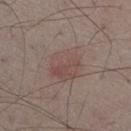{
  "biopsy_status": "not biopsied; imaged during a skin examination",
  "lighting": "white-light",
  "image": {
    "source": "total-body photography crop",
    "field_of_view_mm": 15
  },
  "lesion_size": {
    "long_diameter_mm_approx": 4.5
  },
  "patient": {
    "sex": "male",
    "age_approx": 75
  },
  "site": "right thigh",
  "automated_metrics": {
    "area_mm2_approx": 13.0,
    "shape_asymmetry": 0.15,
    "cielab_L": 48,
    "cielab_a": 17,
    "cielab_b": 21,
    "vs_skin_darker_L": 6.0,
    "vs_skin_contrast_norm": 5.0,
    "border_irregularity_0_10": 1.5,
    "color_variation_0_10": 4.0
  }
}